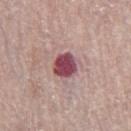{"biopsy_status": "not biopsied; imaged during a skin examination", "patient": {"sex": "female", "age_approx": 65}, "site": "leg", "image": {"source": "total-body photography crop", "field_of_view_mm": 15}}